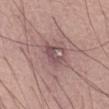Assessment:
Recorded during total-body skin imaging; not selected for excision or biopsy.
Image and clinical context:
A 15 mm close-up tile from a total-body photography series done for melanoma screening. A male subject roughly 75 years of age. Approximately 4 mm at its widest. From the abdomen. Imaged with white-light lighting.